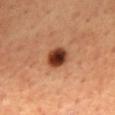| key | value |
|---|---|
| notes | imaged on a skin check; not biopsied |
| anatomic site | the back |
| patient | male, approximately 65 years of age |
| acquisition | 15 mm crop, total-body photography |